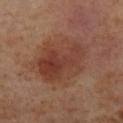The lesion was photographed on a routine skin check and not biopsied; there is no pathology result. A region of skin cropped from a whole-body photographic capture, roughly 15 mm wide. The lesion is on the left lower leg. A female patient, about 55 years old.